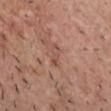<case>
<biopsy_status>not biopsied; imaged during a skin examination</biopsy_status>
<patient>
  <sex>male</sex>
  <age_approx>40</age_approx>
</patient>
<lesion_size>
  <long_diameter_mm_approx>2.5</long_diameter_mm_approx>
</lesion_size>
<image>
  <source>total-body photography crop</source>
  <field_of_view_mm>15</field_of_view_mm>
</image>
<automated_metrics>
  <area_mm2_approx>3.5</area_mm2_approx>
  <eccentricity>0.85</eccentricity>
  <shape_asymmetry>0.35</shape_asymmetry>
  <cielab_L>51</cielab_L>
  <cielab_a>22</cielab_a>
  <cielab_b>27</cielab_b>
  <vs_skin_darker_L>6.0</vs_skin_darker_L>
  <vs_skin_contrast_norm>5.0</vs_skin_contrast_norm>
  <border_irregularity_0_10>3.5</border_irregularity_0_10>
  <lesion_detection_confidence_0_100>100</lesion_detection_confidence_0_100>
</automated_metrics>
<site>head or neck</site>
<lighting>white-light</lighting>
</case>